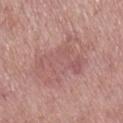Case summary:
– image source: ~15 mm crop, total-body skin-cancer survey
– illumination: white-light illumination
– patient: female, aged 68 to 72
– anatomic site: the leg